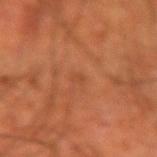Clinical impression: No biopsy was performed on this lesion — it was imaged during a full skin examination and was not determined to be concerning. Acquisition and patient details: The lesion-visualizer software estimated a color-variation rating of about 0/10 and a peripheral color-asymmetry measure near 0. It also reported a classifier nevus-likeness of about 0/100 and a detector confidence of about 100 out of 100 that the crop contains a lesion. On the left forearm. The patient is a male approximately 55 years of age. Approximately 1.5 mm at its widest. This is a cross-polarized tile. Cropped from a total-body skin-imaging series; the visible field is about 15 mm.